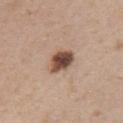| feature | finding |
|---|---|
| workup | no biopsy performed (imaged during a skin exam) |
| image source | ~15 mm crop, total-body skin-cancer survey |
| automated lesion analysis | an average lesion color of about L≈48 a*≈19 b*≈27 (CIELAB), roughly 18 lightness units darker than nearby skin, and a normalized border contrast of about 12; internal color variation of about 6.5 on a 0–10 scale and peripheral color asymmetry of about 2 |
| site | the chest |
| size | ~3.5 mm (longest diameter) |
| subject | male, about 50 years old |
| lighting | white-light |